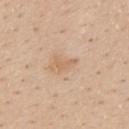Assessment:
Recorded during total-body skin imaging; not selected for excision or biopsy.